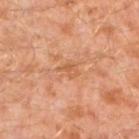  site: left lower leg
  patient:
    sex: male
    age_approx: 30
  image:
    source: total-body photography crop
    field_of_view_mm: 15